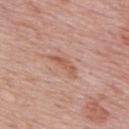Clinical impression: Captured during whole-body skin photography for melanoma surveillance; the lesion was not biopsied. Context: Measured at roughly 4 mm in maximum diameter. Located on the upper back. A male patient, about 75 years old. A 15 mm crop from a total-body photograph taken for skin-cancer surveillance.